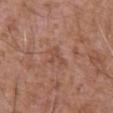The lesion was photographed on a routine skin check and not biopsied; there is no pathology result.
From the abdomen.
This is a white-light tile.
Longest diameter approximately 2.5 mm.
The subject is a male roughly 75 years of age.
A roughly 15 mm field-of-view crop from a total-body skin photograph.